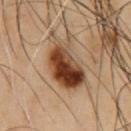{
  "biopsy_status": "not biopsied; imaged during a skin examination",
  "automated_metrics": {
    "area_mm2_approx": 19.0,
    "eccentricity": 0.8,
    "shape_asymmetry": 0.25,
    "border_irregularity_0_10": 3.5,
    "color_variation_0_10": 9.5,
    "peripheral_color_asymmetry": 4.0
  },
  "site": "chest",
  "image": {
    "source": "total-body photography crop",
    "field_of_view_mm": 15
  },
  "lesion_size": {
    "long_diameter_mm_approx": 7.0
  },
  "patient": {
    "sex": "male",
    "age_approx": 55
  },
  "lighting": "cross-polarized"
}